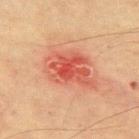<case>
  <biopsy_status>not biopsied; imaged during a skin examination</biopsy_status>
  <patient>
    <sex>male</sex>
    <age_approx>75</age_approx>
  </patient>
  <image>
    <source>total-body photography crop</source>
    <field_of_view_mm>15</field_of_view_mm>
  </image>
  <automated_metrics>
    <vs_skin_darker_L>10.0</vs_skin_darker_L>
    <vs_skin_contrast_norm>7.5</vs_skin_contrast_norm>
    <border_irregularity_0_10>3.5</border_irregularity_0_10>
    <peripheral_color_asymmetry>2.5</peripheral_color_asymmetry>
    <nevus_likeness_0_100>0</nevus_likeness_0_100>
    <lesion_detection_confidence_0_100>100</lesion_detection_confidence_0_100>
  </automated_metrics>
  <site>upper back</site>
  <lesion_size>
    <long_diameter_mm_approx>6.0</long_diameter_mm_approx>
  </lesion_size>
</case>